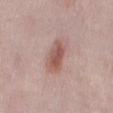Image and clinical context: On the mid back. A female patient, roughly 40 years of age. A roughly 15 mm field-of-view crop from a total-body skin photograph. This is a white-light tile.